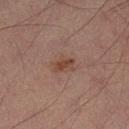A male patient, in their 30s. Approximately 3 mm at its widest. The lesion is on the left lower leg. The total-body-photography lesion software estimated an outline eccentricity of about 0.8 (0 = round, 1 = elongated) and a shape-asymmetry score of about 0.35 (0 = symmetric). And it measured an average lesion color of about L≈35 a*≈16 b*≈23 (CIELAB), about 6 CIELAB-L* units darker than the surrounding skin, and a normalized border contrast of about 7. This is a cross-polarized tile. A roughly 15 mm field-of-view crop from a total-body skin photograph.